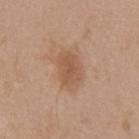Acquisition and patient details:
About 4 mm across. A lesion tile, about 15 mm wide, cut from a 3D total-body photograph. A female subject in their 40s. From the left upper arm.